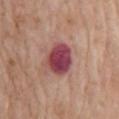follow-up: total-body-photography surveillance lesion; no biopsy | imaging modality: ~15 mm tile from a whole-body skin photo | location: the mid back | lighting: white-light | lesion diameter: ~4 mm (longest diameter) | patient: female, in their mid-70s.